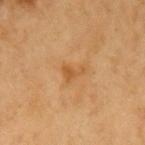Q: Is there a histopathology result?
A: imaged on a skin check; not biopsied
Q: What kind of image is this?
A: total-body-photography crop, ~15 mm field of view
Q: What are the patient's age and sex?
A: male, aged 58 to 62
Q: What is the anatomic site?
A: the arm